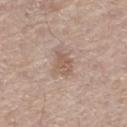workup=catalogued during a skin exam; not biopsied